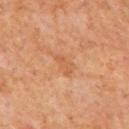Clinical impression:
This lesion was catalogued during total-body skin photography and was not selected for biopsy.
Acquisition and patient details:
Cropped from a whole-body photographic skin survey; the tile spans about 15 mm. This is a cross-polarized tile. A male subject aged 63–67. The lesion is located on the mid back.